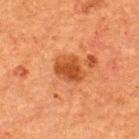Recorded during total-body skin imaging; not selected for excision or biopsy.
A 15 mm close-up extracted from a 3D total-body photography capture.
From the upper back.
The subject is a male aged approximately 50.
Captured under cross-polarized illumination.